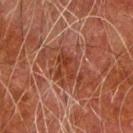Q: What kind of image is this?
A: 15 mm crop, total-body photography
Q: Who is the patient?
A: male, approximately 80 years of age
Q: Where on the body is the lesion?
A: the right upper arm